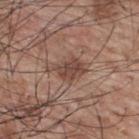  site: back
  lighting: white-light
  lesion_size:
    long_diameter_mm_approx: 4.0
  patient:
    sex: male
    age_approx: 70
  image:
    source: total-body photography crop
    field_of_view_mm: 15